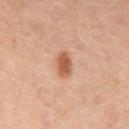Part of a total-body skin-imaging series; this lesion was reviewed on a skin check and was not flagged for biopsy.
A close-up tile cropped from a whole-body skin photograph, about 15 mm across.
The subject is a male in their 60s.
Located on the abdomen.